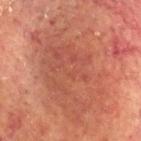| feature | finding |
|---|---|
| biopsy status | no biopsy performed (imaged during a skin exam) |
| patient | male, aged approximately 55 |
| location | the head or neck |
| imaging modality | total-body-photography crop, ~15 mm field of view |